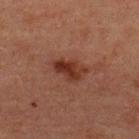This lesion was catalogued during total-body skin photography and was not selected for biopsy.
The lesion is on the upper back.
This is a cross-polarized tile.
About 3.5 mm across.
The patient is a male aged around 50.
The lesion-visualizer software estimated an area of roughly 7 mm², a shape eccentricity near 0.8, and a symmetry-axis asymmetry near 0.3. It also reported a border-irregularity index near 3/10, internal color variation of about 4.5 on a 0–10 scale, and peripheral color asymmetry of about 1.5. The software also gave a nevus-likeness score of about 70/100 and a detector confidence of about 100 out of 100 that the crop contains a lesion.
A close-up tile cropped from a whole-body skin photograph, about 15 mm across.